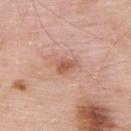<tbp_lesion>
<biopsy_status>not biopsied; imaged during a skin examination</biopsy_status>
<patient>
  <sex>male</sex>
  <age_approx>55</age_approx>
</patient>
<site>upper back</site>
<image>
  <source>total-body photography crop</source>
  <field_of_view_mm>15</field_of_view_mm>
</image>
<automated_metrics>
  <area_mm2_approx>4.0</area_mm2_approx>
  <eccentricity>0.75</eccentricity>
  <shape_asymmetry>0.3</shape_asymmetry>
  <border_irregularity_0_10>3.0</border_irregularity_0_10>
  <color_variation_0_10>2.0</color_variation_0_10>
  <peripheral_color_asymmetry>0.5</peripheral_color_asymmetry>
</automated_metrics>
<lighting>white-light</lighting>
</tbp_lesion>